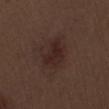Case summary:
* workup · imaged on a skin check; not biopsied
* imaging modality · 15 mm crop, total-body photography
* patient · male, aged 68–72
* anatomic site · the right upper arm